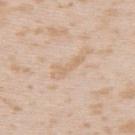notes — total-body-photography surveillance lesion; no biopsy
location — the upper back
image — total-body-photography crop, ~15 mm field of view
diameter — about 4 mm
lighting — white-light
automated lesion analysis — a lesion color around L≈68 a*≈15 b*≈32 in CIELAB, roughly 6 lightness units darker than nearby skin, and a lesion-to-skin contrast of about 5 (normalized; higher = more distinct); a border-irregularity rating of about 6.5/10, a color-variation rating of about 0/10, and peripheral color asymmetry of about 0
subject — female, roughly 25 years of age